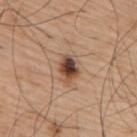Impression:
Part of a total-body skin-imaging series; this lesion was reviewed on a skin check and was not flagged for biopsy.
Acquisition and patient details:
Located on the upper back. Cropped from a total-body skin-imaging series; the visible field is about 15 mm. Automated image analysis of the tile measured a detector confidence of about 100 out of 100 that the crop contains a lesion. A male patient about 75 years old. Captured under white-light illumination.